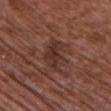Acquisition and patient details:
The lesion is on the chest. The patient is a male aged 73 to 77. A close-up tile cropped from a whole-body skin photograph, about 15 mm across. An algorithmic analysis of the crop reported an eccentricity of roughly 0.8 and a shape-asymmetry score of about 0.35 (0 = symmetric). And it measured a mean CIELAB color near L≈33 a*≈20 b*≈23, roughly 7 lightness units darker than nearby skin, and a lesion-to-skin contrast of about 7 (normalized; higher = more distinct). And it measured a border-irregularity index near 4/10, a within-lesion color-variation index near 3.5/10, and peripheral color asymmetry of about 1. It also reported a nevus-likeness score of about 0/100.